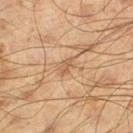Acquisition and patient details:
Automated image analysis of the tile measured internal color variation of about 0 on a 0–10 scale and peripheral color asymmetry of about 0. The analysis additionally found a classifier nevus-likeness of about 0/100 and lesion-presence confidence of about 85/100. A lesion tile, about 15 mm wide, cut from a 3D total-body photograph. Longest diameter approximately 2.5 mm. This is a cross-polarized tile. On the left thigh. The patient is a male about 60 years old.